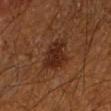| feature | finding |
|---|---|
| workup | no biopsy performed (imaged during a skin exam) |
| anatomic site | the left forearm |
| lesion size | ~4 mm (longest diameter) |
| tile lighting | cross-polarized |
| image source | 15 mm crop, total-body photography |
| patient | male, approximately 60 years of age |